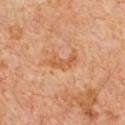notes: total-body-photography surveillance lesion; no biopsy
subject: male, in their 60s
acquisition: 15 mm crop, total-body photography
site: the front of the torso